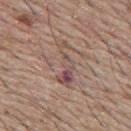{"biopsy_status": "not biopsied; imaged during a skin examination", "image": {"source": "total-body photography crop", "field_of_view_mm": 15}, "lighting": "white-light", "patient": {"sex": "male", "age_approx": 65}, "site": "back", "lesion_size": {"long_diameter_mm_approx": 6.0}, "automated_metrics": {"area_mm2_approx": 8.5, "eccentricity": 0.95, "shape_asymmetry": 0.45}}